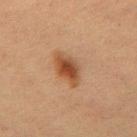This lesion was catalogued during total-body skin photography and was not selected for biopsy.